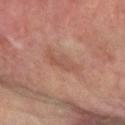Assessment:
The lesion was photographed on a routine skin check and not biopsied; there is no pathology result.